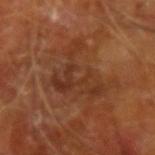Impression: No biopsy was performed on this lesion — it was imaged during a full skin examination and was not determined to be concerning. Image and clinical context: The lesion is on the left forearm. The total-body-photography lesion software estimated a lesion area of about 16 mm², an outline eccentricity of about 0.5 (0 = round, 1 = elongated), and two-axis asymmetry of about 0.7. And it measured an average lesion color of about L≈33 a*≈22 b*≈30 (CIELAB), roughly 6 lightness units darker than nearby skin, and a normalized lesion–skin contrast near 6. The analysis additionally found a border-irregularity rating of about 10/10 and peripheral color asymmetry of about 1.5. The analysis additionally found an automated nevus-likeness rating near 0 out of 100 and a lesion-detection confidence of about 90/100. A male subject, roughly 65 years of age. A roughly 15 mm field-of-view crop from a total-body skin photograph. Captured under cross-polarized illumination.